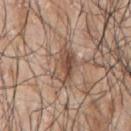workup: imaged on a skin check; not biopsied
lighting: white-light illumination
location: the left arm
patient: male, in their 70s
acquisition: ~15 mm tile from a whole-body skin photo